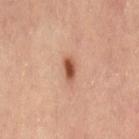Part of a total-body skin-imaging series; this lesion was reviewed on a skin check and was not flagged for biopsy.
The lesion's longest dimension is about 3 mm.
A 15 mm close-up extracted from a 3D total-body photography capture.
The lesion is on the left thigh.
The lesion-visualizer software estimated an area of roughly 4 mm², an eccentricity of roughly 0.85, and two-axis asymmetry of about 0.2. And it measured a lesion color around L≈53 a*≈25 b*≈33 in CIELAB, roughly 15 lightness units darker than nearby skin, and a normalized lesion–skin contrast near 10.
Captured under cross-polarized illumination.
A female patient aged 33 to 37.